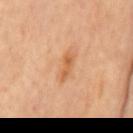follow-up=total-body-photography surveillance lesion; no biopsy